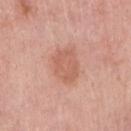Notes:
• notes: catalogued during a skin exam; not biopsied
• imaging modality: ~15 mm crop, total-body skin-cancer survey
• anatomic site: the arm
• lesion size: ~4.5 mm (longest diameter)
• subject: female, in their mid- to late 60s
• illumination: white-light